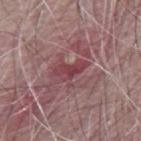notes: catalogued during a skin exam; not biopsied | body site: the mid back | illumination: white-light illumination | subject: male, aged 63 to 67 | acquisition: total-body-photography crop, ~15 mm field of view.